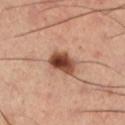This lesion was catalogued during total-body skin photography and was not selected for biopsy. The lesion's longest dimension is about 3.5 mm. Cropped from a whole-body photographic skin survey; the tile spans about 15 mm. Captured under cross-polarized illumination. The patient is a male about 65 years old. Automated image analysis of the tile measured a lesion area of about 8 mm², an eccentricity of roughly 0.55, and a shape-asymmetry score of about 0.2 (0 = symmetric). And it measured a lesion color around L≈46 a*≈23 b*≈30 in CIELAB, roughly 17 lightness units darker than nearby skin, and a normalized lesion–skin contrast near 12. The analysis additionally found lesion-presence confidence of about 100/100. Located on the leg.